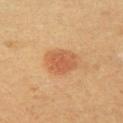Q: Was a biopsy performed?
A: no biopsy performed (imaged during a skin exam)
Q: What is the anatomic site?
A: the left upper arm
Q: Automated lesion metrics?
A: an average lesion color of about L≈51 a*≈22 b*≈35 (CIELAB)
Q: How large is the lesion?
A: about 4 mm
Q: Patient demographics?
A: male, aged around 40
Q: What is the imaging modality?
A: ~15 mm tile from a whole-body skin photo